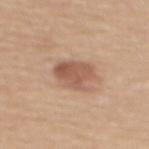| feature | finding |
|---|---|
| notes | imaged on a skin check; not biopsied |
| subject | female, in their mid- to late 50s |
| tile lighting | white-light illumination |
| acquisition | 15 mm crop, total-body photography |
| site | the upper back |
| lesion size | ~4 mm (longest diameter) |